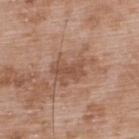follow-up = total-body-photography surveillance lesion; no biopsy
subject = male, in their 50s
acquisition = ~15 mm tile from a whole-body skin photo
TBP lesion metrics = a footprint of about 11 mm² and two-axis asymmetry of about 0.35; a detector confidence of about 100 out of 100 that the crop contains a lesion
location = the upper back